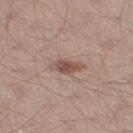Notes:
• notes: imaged on a skin check; not biopsied
• diameter: about 3.5 mm
• subject: male, in their mid- to late 30s
• image source: ~15 mm crop, total-body skin-cancer survey
• TBP lesion metrics: a border-irregularity rating of about 3/10 and peripheral color asymmetry of about 1.5; an automated nevus-likeness rating near 70 out of 100 and lesion-presence confidence of about 100/100
• location: the right thigh
• lighting: white-light